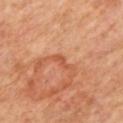Clinical summary: The subject is aged 63–67. A 15 mm close-up extracted from a 3D total-body photography capture. Located on the mid back.